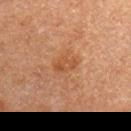The lesion was tiled from a total-body skin photograph and was not biopsied.
An algorithmic analysis of the crop reported a footprint of about 4 mm² and two-axis asymmetry of about 0.3. It also reported a border-irregularity index near 3/10, a color-variation rating of about 3/10, and a peripheral color-asymmetry measure near 1.
The lesion is located on the left upper arm.
A 15 mm close-up extracted from a 3D total-body photography capture.
The lesion's longest dimension is about 3 mm.
A female subject, roughly 55 years of age.
This is a cross-polarized tile.